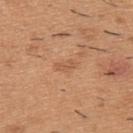biopsy status — no biopsy performed (imaged during a skin exam) | body site — the upper back | patient — male, aged approximately 40 | lesion diameter — ≈3 mm | tile lighting — white-light illumination | imaging modality — total-body-photography crop, ~15 mm field of view.